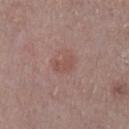{
  "biopsy_status": "not biopsied; imaged during a skin examination",
  "patient": {
    "sex": "male",
    "age_approx": 70
  },
  "lighting": "white-light",
  "image": {
    "source": "total-body photography crop",
    "field_of_view_mm": 15
  },
  "lesion_size": {
    "long_diameter_mm_approx": 3.0
  },
  "automated_metrics": {
    "eccentricity": 0.8,
    "shape_asymmetry": 0.25,
    "color_variation_0_10": 1.5,
    "peripheral_color_asymmetry": 0.5
  },
  "site": "left lower leg"
}